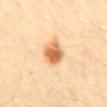Part of a total-body skin-imaging series; this lesion was reviewed on a skin check and was not flagged for biopsy. A male subject aged 38–42. Automated image analysis of the tile measured a border-irregularity index near 2/10 and radial color variation of about 2. A 15 mm close-up extracted from a 3D total-body photography capture. This is a cross-polarized tile. On the abdomen.